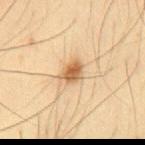Findings:
– follow-up: total-body-photography surveillance lesion; no biopsy
– automated lesion analysis: a lesion–skin lightness drop of about 13 and a normalized border contrast of about 9; border irregularity of about 2.5 on a 0–10 scale, a color-variation rating of about 4/10, and a peripheral color-asymmetry measure near 1.5
– diameter: ~3 mm (longest diameter)
– tile lighting: cross-polarized illumination
– subject: male, about 35 years old
– body site: the back
– imaging modality: total-body-photography crop, ~15 mm field of view